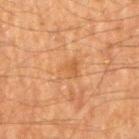Recorded during total-body skin imaging; not selected for excision or biopsy. An algorithmic analysis of the crop reported a footprint of about 5.5 mm², an outline eccentricity of about 0.75 (0 = round, 1 = elongated), and a symmetry-axis asymmetry near 0.25. It also reported roughly 5 lightness units darker than nearby skin and a normalized border contrast of about 4.5. The lesion is located on the right upper arm. The patient is a male in their mid- to late 60s. This image is a 15 mm lesion crop taken from a total-body photograph. Captured under cross-polarized illumination.